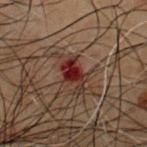This lesion was catalogued during total-body skin photography and was not selected for biopsy.
The patient is a male aged 48 to 52.
The lesion is on the chest.
A 15 mm close-up extracted from a 3D total-body photography capture.
About 3.5 mm across.